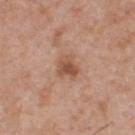Impression:
Recorded during total-body skin imaging; not selected for excision or biopsy.
Image and clinical context:
A close-up tile cropped from a whole-body skin photograph, about 15 mm across. From the chest. The patient is a male about 50 years old. Automated image analysis of the tile measured a color-variation rating of about 4.5/10 and peripheral color asymmetry of about 1.5. This is a white-light tile. The recorded lesion diameter is about 2.5 mm.